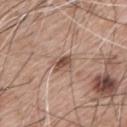  biopsy_status: not biopsied; imaged during a skin examination
  lesion_size:
    long_diameter_mm_approx: 3.0
  automated_metrics:
    area_mm2_approx: 5.0
    eccentricity: 0.75
    cielab_L: 52
    cielab_a: 18
    cielab_b: 26
    vs_skin_darker_L: 11.0
    vs_skin_contrast_norm: 7.5
    lesion_detection_confidence_0_100: 100
  image:
    source: total-body photography crop
    field_of_view_mm: 15
  lighting: white-light
  patient:
    sex: male
    age_approx: 60
  site: mid back